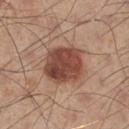This lesion was catalogued during total-body skin photography and was not selected for biopsy. The total-body-photography lesion software estimated a border-irregularity rating of about 1.5/10, internal color variation of about 5 on a 0–10 scale, and a peripheral color-asymmetry measure near 1.5. A lesion tile, about 15 mm wide, cut from a 3D total-body photograph. The subject is a male aged 53–57. The lesion is located on the left lower leg. Approximately 5 mm at its widest.